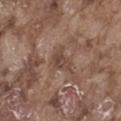No biopsy was performed on this lesion — it was imaged during a full skin examination and was not determined to be concerning. Captured under white-light illumination. A male subject about 75 years old. This image is a 15 mm lesion crop taken from a total-body photograph. From the leg. Approximately 3 mm at its widest.